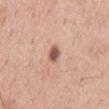Q: Was this lesion biopsied?
A: imaged on a skin check; not biopsied
Q: What did automated image analysis measure?
A: a symmetry-axis asymmetry near 0.25
Q: What is the imaging modality?
A: ~15 mm tile from a whole-body skin photo
Q: How large is the lesion?
A: ~2.5 mm (longest diameter)
Q: Who is the patient?
A: male, aged around 60
Q: What is the anatomic site?
A: the mid back
Q: What lighting was used for the tile?
A: white-light illumination